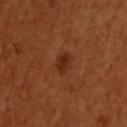| field | value |
|---|---|
| workup | no biopsy performed (imaged during a skin exam) |
| diameter | about 2.5 mm |
| acquisition | ~15 mm crop, total-body skin-cancer survey |
| illumination | cross-polarized |
| automated metrics | an outline eccentricity of about 0.65 (0 = round, 1 = elongated) and a symmetry-axis asymmetry near 0.2; a normalized lesion–skin contrast near 7.5; a border-irregularity rating of about 2/10, a color-variation rating of about 1.5/10, and radial color variation of about 0.5; a classifier nevus-likeness of about 80/100 |
| patient | male, in their 60s |
| site | the upper back |